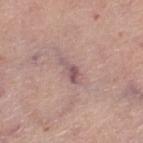patient: female, aged 63 to 67
imaging modality: 15 mm crop, total-body photography
illumination: white-light
automated metrics: a lesion area of about 4 mm² and a symmetry-axis asymmetry near 0.35; a nevus-likeness score of about 0/100
body site: the right lower leg